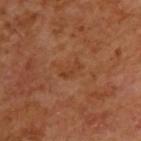| feature | finding |
|---|---|
| automated lesion analysis | a lesion area of about 2.5 mm², an outline eccentricity of about 0.9 (0 = round, 1 = elongated), and a shape-asymmetry score of about 0.4 (0 = symmetric); an automated nevus-likeness rating near 0 out of 100 |
| image source | total-body-photography crop, ~15 mm field of view |
| diameter | ≈2.5 mm |
| lighting | cross-polarized |
| site | the upper back |
| subject | male, about 65 years old |